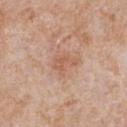<tbp_lesion>
<biopsy_status>not biopsied; imaged during a skin examination</biopsy_status>
<lighting>white-light</lighting>
<site>chest</site>
<automated_metrics>
  <nevus_likeness_0_100>0</nevus_likeness_0_100>
  <lesion_detection_confidence_0_100>100</lesion_detection_confidence_0_100>
</automated_metrics>
<lesion_size>
  <long_diameter_mm_approx>3.0</long_diameter_mm_approx>
</lesion_size>
<image>
  <source>total-body photography crop</source>
  <field_of_view_mm>15</field_of_view_mm>
</image>
<patient>
  <sex>male</sex>
  <age_approx>65</age_approx>
</patient>
</tbp_lesion>